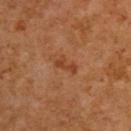Captured during whole-body skin photography for melanoma surveillance; the lesion was not biopsied. A roughly 15 mm field-of-view crop from a total-body skin photograph. The lesion's longest dimension is about 2.5 mm. The subject is a male aged around 60. This is a cross-polarized tile. On the upper back.